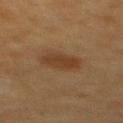Case summary:
– size · ~3.5 mm (longest diameter)
– imaging modality · ~15 mm crop, total-body skin-cancer survey
– subject · female, aged approximately 55
– anatomic site · the upper back
– tile lighting · cross-polarized
– image-analysis metrics · a mean CIELAB color near L≈32 a*≈16 b*≈28, about 7 CIELAB-L* units darker than the surrounding skin, and a normalized lesion–skin contrast near 7; a border-irregularity rating of about 1.5/10, internal color variation of about 2 on a 0–10 scale, and a peripheral color-asymmetry measure near 0.5; an automated nevus-likeness rating near 95 out of 100 and lesion-presence confidence of about 100/100